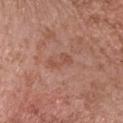Q: Was this lesion biopsied?
A: total-body-photography surveillance lesion; no biopsy
Q: What kind of image is this?
A: ~15 mm crop, total-body skin-cancer survey
Q: Lesion size?
A: about 3 mm
Q: What did automated image analysis measure?
A: border irregularity of about 6 on a 0–10 scale, a within-lesion color-variation index near 0/10, and peripheral color asymmetry of about 0; an automated nevus-likeness rating near 0 out of 100 and a detector confidence of about 100 out of 100 that the crop contains a lesion
Q: Who is the patient?
A: female, about 55 years old
Q: Where on the body is the lesion?
A: the head or neck